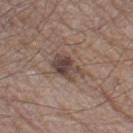| key | value |
|---|---|
| biopsy status | imaged on a skin check; not biopsied |